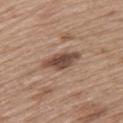{"biopsy_status": "not biopsied; imaged during a skin examination", "lesion_size": {"long_diameter_mm_approx": 5.0}, "patient": {"sex": "female", "age_approx": 60}, "site": "upper back", "lighting": "white-light", "image": {"source": "total-body photography crop", "field_of_view_mm": 15}}